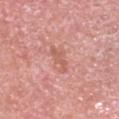workup = total-body-photography surveillance lesion; no biopsy | lesion diameter = ~3.5 mm (longest diameter) | anatomic site = the head or neck | TBP lesion metrics = a footprint of about 4 mm², an eccentricity of roughly 0.9, and a symmetry-axis asymmetry near 0.4; a mean CIELAB color near L≈59 a*≈27 b*≈29, about 7 CIELAB-L* units darker than the surrounding skin, and a normalized border contrast of about 5.5; a border-irregularity rating of about 5.5/10, a within-lesion color-variation index near 0.5/10, and peripheral color asymmetry of about 0 | imaging modality = total-body-photography crop, ~15 mm field of view | patient = male, aged 68 to 72.